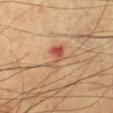A close-up tile cropped from a whole-body skin photograph, about 15 mm across. Located on the leg. The subject is a male roughly 70 years of age.